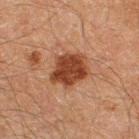<case>
  <patient>
    <sex>male</sex>
    <age_approx>60</age_approx>
  </patient>
  <lighting>cross-polarized</lighting>
  <automated_metrics>
    <border_irregularity_0_10>2.5</border_irregularity_0_10>
    <peripheral_color_asymmetry>1.0</peripheral_color_asymmetry>
  </automated_metrics>
  <site>right thigh</site>
  <image>
    <source>total-body photography crop</source>
    <field_of_view_mm>15</field_of_view_mm>
  </image>
</case>